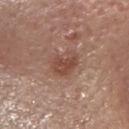notes: total-body-photography surveillance lesion; no biopsy
diameter: ~3 mm (longest diameter)
illumination: white-light illumination
image: ~15 mm crop, total-body skin-cancer survey
location: the head or neck
subject: female, in their mid- to late 60s